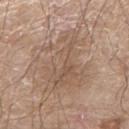Part of a total-body skin-imaging series; this lesion was reviewed on a skin check and was not flagged for biopsy.
The recorded lesion diameter is about 7 mm.
A 15 mm crop from a total-body photograph taken for skin-cancer surveillance.
Automated image analysis of the tile measured an average lesion color of about L≈55 a*≈16 b*≈27 (CIELAB), roughly 7 lightness units darker than nearby skin, and a lesion-to-skin contrast of about 5.5 (normalized; higher = more distinct). The analysis additionally found border irregularity of about 8 on a 0–10 scale and a color-variation rating of about 4.5/10.
The lesion is located on the left arm.
A male subject, aged around 80.
Captured under white-light illumination.